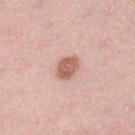notes — no biopsy performed (imaged during a skin exam) | size — ≈3 mm | lighting — white-light | imaging modality — 15 mm crop, total-body photography | subject — female, aged 63–67 | location — the leg | automated lesion analysis — an area of roughly 5.5 mm², an eccentricity of roughly 0.65, and a shape-asymmetry score of about 0.25 (0 = symmetric); an average lesion color of about L≈60 a*≈23 b*≈27 (CIELAB), a lesion–skin lightness drop of about 14, and a lesion-to-skin contrast of about 8.5 (normalized; higher = more distinct); an automated nevus-likeness rating near 95 out of 100 and lesion-presence confidence of about 100/100.